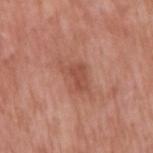This lesion was catalogued during total-body skin photography and was not selected for biopsy. Longest diameter approximately 4 mm. Imaged with white-light lighting. A male subject, in their mid- to late 50s. The lesion is on the right upper arm. A roughly 15 mm field-of-view crop from a total-body skin photograph.